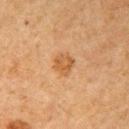Q: Is there a histopathology result?
A: total-body-photography surveillance lesion; no biopsy
Q: How was the tile lit?
A: cross-polarized illumination
Q: Patient demographics?
A: female, aged around 60
Q: Lesion location?
A: the right upper arm
Q: What is the imaging modality?
A: ~15 mm tile from a whole-body skin photo